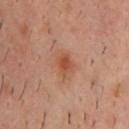This lesion was catalogued during total-body skin photography and was not selected for biopsy.
Imaged with cross-polarized lighting.
The patient is a male aged around 40.
Cropped from a whole-body photographic skin survey; the tile spans about 15 mm.
An algorithmic analysis of the crop reported a mean CIELAB color near L≈50 a*≈23 b*≈32, about 8 CIELAB-L* units darker than the surrounding skin, and a lesion-to-skin contrast of about 6.5 (normalized; higher = more distinct). The analysis additionally found a classifier nevus-likeness of about 50/100.
The lesion is on the back.
About 3.5 mm across.